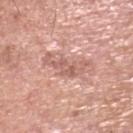Captured during whole-body skin photography for melanoma surveillance; the lesion was not biopsied. On the head or neck. A 15 mm close-up tile from a total-body photography series done for melanoma screening. Automated image analysis of the tile measured an average lesion color of about L≈60 a*≈23 b*≈26 (CIELAB), roughly 9 lightness units darker than nearby skin, and a normalized border contrast of about 6. The analysis additionally found a classifier nevus-likeness of about 0/100 and lesion-presence confidence of about 95/100. A male patient aged 58–62.